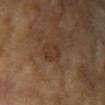No biopsy was performed on this lesion — it was imaged during a full skin examination and was not determined to be concerning.